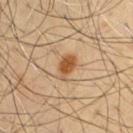No biopsy was performed on this lesion — it was imaged during a full skin examination and was not determined to be concerning.
A 15 mm close-up tile from a total-body photography series done for melanoma screening.
A male patient, in their mid- to late 50s.
On the front of the torso.